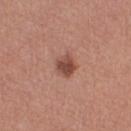Recorded during total-body skin imaging; not selected for excision or biopsy. From the right thigh. A female subject approximately 60 years of age. A roughly 15 mm field-of-view crop from a total-body skin photograph. Approximately 2.5 mm at its widest. Captured under white-light illumination.